A close-up tile cropped from a whole-body skin photograph, about 15 mm across; on the leg; a female subject, aged around 20:
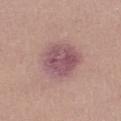{
  "lighting": "white-light",
  "lesion_size": {
    "long_diameter_mm_approx": 5.5
  },
  "diagnosis": {
    "histopathology": "dysplastic (Clark) nevus",
    "malignancy": "benign",
    "taxonomic_path": [
      "Benign",
      "Benign melanocytic proliferations",
      "Nevus",
      "Nevus, Atypical, Dysplastic, or Clark"
    ]
  }
}